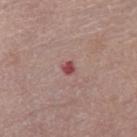<record>
<biopsy_status>not biopsied; imaged during a skin examination</biopsy_status>
<automated_metrics>
  <area_mm2_approx>2.0</area_mm2_approx>
  <shape_asymmetry>0.3</shape_asymmetry>
  <nevus_likeness_0_100>0</nevus_likeness_0_100>
  <lesion_detection_confidence_0_100>100</lesion_detection_confidence_0_100>
</automated_metrics>
<site>leg</site>
<lesion_size>
  <long_diameter_mm_approx>1.5</long_diameter_mm_approx>
</lesion_size>
<image>
  <source>total-body photography crop</source>
  <field_of_view_mm>15</field_of_view_mm>
</image>
<lighting>white-light</lighting>
<patient>
  <sex>female</sex>
  <age_approx>65</age_approx>
</patient>
</record>